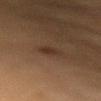The lesion's longest dimension is about 2.5 mm. The patient is a male aged 83 or over. From the arm. Automated image analysis of the tile measured a symmetry-axis asymmetry near 0.25. It also reported a mean CIELAB color near L≈28 a*≈14 b*≈23, a lesion–skin lightness drop of about 6, and a lesion-to-skin contrast of about 6.5 (normalized; higher = more distinct). A region of skin cropped from a whole-body photographic capture, roughly 15 mm wide.